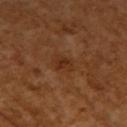notes: catalogued during a skin exam; not biopsied
illumination: cross-polarized
diameter: ≈3 mm
subject: female, roughly 55 years of age
automated lesion analysis: a mean CIELAB color near L≈32 a*≈22 b*≈33 and a normalized lesion–skin contrast near 6.5
imaging modality: ~15 mm crop, total-body skin-cancer survey
location: the right upper arm